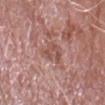- workup — total-body-photography surveillance lesion; no biopsy
- image — total-body-photography crop, ~15 mm field of view
- subject — male, aged 73–77
- lighting — white-light
- location — the right forearm